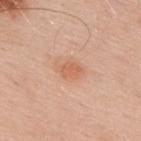Assessment: The lesion was photographed on a routine skin check and not biopsied; there is no pathology result. Acquisition and patient details: The recorded lesion diameter is about 3 mm. Cropped from a total-body skin-imaging series; the visible field is about 15 mm. This is a white-light tile. The lesion is located on the upper back. A male patient in their mid- to late 50s.